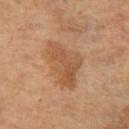Clinical impression: The lesion was photographed on a routine skin check and not biopsied; there is no pathology result. Context: Measured at roughly 6.5 mm in maximum diameter. On the left thigh. An algorithmic analysis of the crop reported a border-irregularity index near 4/10, a within-lesion color-variation index near 3.5/10, and a peripheral color-asymmetry measure near 1.5. And it measured a classifier nevus-likeness of about 20/100. A 15 mm close-up extracted from a 3D total-body photography capture. The subject is a female aged 53 to 57.